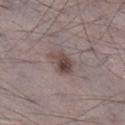Q: Was a biopsy performed?
A: imaged on a skin check; not biopsied